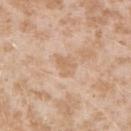Assessment: The lesion was tiled from a total-body skin photograph and was not biopsied. Clinical summary: The patient is a female aged around 25. The lesion's longest dimension is about 3 mm. A close-up tile cropped from a whole-body skin photograph, about 15 mm across. On the right upper arm. Automated tile analysis of the lesion measured a footprint of about 4.5 mm², an eccentricity of roughly 0.65, and a shape-asymmetry score of about 0.4 (0 = symmetric). The analysis additionally found a border-irregularity index near 4.5/10, a within-lesion color-variation index near 1.5/10, and peripheral color asymmetry of about 0.5. Captured under white-light illumination.